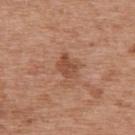Impression: No biopsy was performed on this lesion — it was imaged during a full skin examination and was not determined to be concerning. Acquisition and patient details: This image is a 15 mm lesion crop taken from a total-body photograph. A female patient aged around 40. From the upper back. Automated image analysis of the tile measured a lesion area of about 5.5 mm² and a shape-asymmetry score of about 0.35 (0 = symmetric). It also reported border irregularity of about 3.5 on a 0–10 scale, internal color variation of about 2 on a 0–10 scale, and peripheral color asymmetry of about 1. It also reported a classifier nevus-likeness of about 0/100 and a detector confidence of about 100 out of 100 that the crop contains a lesion. About 3 mm across.